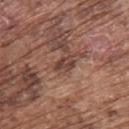Imaged during a routine full-body skin examination; the lesion was not biopsied and no histopathology is available. An algorithmic analysis of the crop reported an area of roughly 3 mm² and a shape-asymmetry score of about 0.3 (0 = symmetric). It also reported a lesion color around L≈41 a*≈20 b*≈24 in CIELAB, roughly 10 lightness units darker than nearby skin, and a normalized border contrast of about 8.5. The software also gave a color-variation rating of about 2.5/10. The subject is a male in their mid- to late 70s. A region of skin cropped from a whole-body photographic capture, roughly 15 mm wide. The tile uses white-light illumination. The lesion is located on the back.